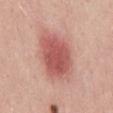No biopsy was performed on this lesion — it was imaged during a full skin examination and was not determined to be concerning. Longest diameter approximately 6.5 mm. A male subject aged 23 to 27. The total-body-photography lesion software estimated an area of roughly 22 mm², a shape eccentricity near 0.7, and a shape-asymmetry score of about 0.15 (0 = symmetric). It also reported lesion-presence confidence of about 100/100. Located on the mid back. Cropped from a total-body skin-imaging series; the visible field is about 15 mm.